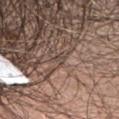No biopsy was performed on this lesion — it was imaged during a full skin examination and was not determined to be concerning. A female subject approximately 50 years of age. A 15 mm close-up extracted from a 3D total-body photography capture. From the head or neck.